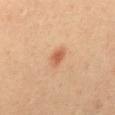Clinical impression:
Part of a total-body skin-imaging series; this lesion was reviewed on a skin check and was not flagged for biopsy.
Clinical summary:
A male subject about 40 years old. This is a cross-polarized tile. A roughly 15 mm field-of-view crop from a total-body skin photograph. From the mid back. About 2.5 mm across.